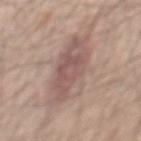Clinical impression:
Captured during whole-body skin photography for melanoma surveillance; the lesion was not biopsied.
Clinical summary:
The subject is a male in their 60s. Located on the mid back. Cropped from a whole-body photographic skin survey; the tile spans about 15 mm. Imaged with white-light lighting.